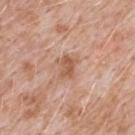Q: Is there a histopathology result?
A: total-body-photography surveillance lesion; no biopsy
Q: Who is the patient?
A: male, aged 48–52
Q: What kind of image is this?
A: ~15 mm crop, total-body skin-cancer survey
Q: Lesion location?
A: the upper back